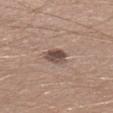No biopsy was performed on this lesion — it was imaged during a full skin examination and was not determined to be concerning. Imaged with white-light lighting. The total-body-photography lesion software estimated a shape eccentricity near 0.65. The analysis additionally found a border-irregularity index near 2/10 and a peripheral color-asymmetry measure near 2. The software also gave a classifier nevus-likeness of about 45/100. From the left lower leg. A male subject, aged 28 to 32. This image is a 15 mm lesion crop taken from a total-body photograph. Longest diameter approximately 3 mm.